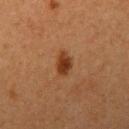Findings:
* workup: imaged on a skin check; not biopsied
* illumination: cross-polarized illumination
* lesion size: about 3 mm
* acquisition: total-body-photography crop, ~15 mm field of view
* site: the right upper arm
* patient: female, aged 38–42